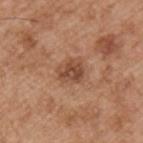Part of a total-body skin-imaging series; this lesion was reviewed on a skin check and was not flagged for biopsy. A region of skin cropped from a whole-body photographic capture, roughly 15 mm wide. A male subject approximately 55 years of age. The lesion's longest dimension is about 3 mm. The lesion-visualizer software estimated an area of roughly 6 mm² and an eccentricity of roughly 0.65. The software also gave an average lesion color of about L≈47 a*≈22 b*≈31 (CIELAB), about 11 CIELAB-L* units darker than the surrounding skin, and a lesion-to-skin contrast of about 8 (normalized; higher = more distinct). And it measured a border-irregularity rating of about 2/10 and internal color variation of about 4 on a 0–10 scale. And it measured an automated nevus-likeness rating near 35 out of 100 and a detector confidence of about 100 out of 100 that the crop contains a lesion. Located on the arm. Captured under white-light illumination.